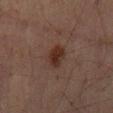– notes · imaged on a skin check; not biopsied
– lesion diameter · ~3 mm (longest diameter)
– patient · male, aged 68–72
– site · the abdomen
– automated lesion analysis · an outline eccentricity of about 0.8 (0 = round, 1 = elongated) and a symmetry-axis asymmetry near 0.25; a lesion–skin lightness drop of about 8 and a lesion-to-skin contrast of about 9.5 (normalized; higher = more distinct); an automated nevus-likeness rating near 90 out of 100
– imaging modality · 15 mm crop, total-body photography
– lighting · cross-polarized illumination